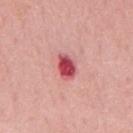Q: Was a biopsy performed?
A: catalogued during a skin exam; not biopsied
Q: What kind of image is this?
A: 15 mm crop, total-body photography
Q: Lesion location?
A: the mid back
Q: What is the lesion's diameter?
A: about 3 mm
Q: Patient demographics?
A: male, aged 63–67